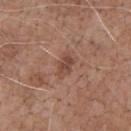Part of a total-body skin-imaging series; this lesion was reviewed on a skin check and was not flagged for biopsy.
Located on the chest.
The tile uses white-light illumination.
The total-body-photography lesion software estimated an area of roughly 4 mm², an outline eccentricity of about 0.8 (0 = round, 1 = elongated), and two-axis asymmetry of about 0.25. The software also gave a border-irregularity rating of about 2.5/10, a within-lesion color-variation index near 2.5/10, and radial color variation of about 1. And it measured a classifier nevus-likeness of about 20/100 and a lesion-detection confidence of about 100/100.
A male subject aged 68–72.
A roughly 15 mm field-of-view crop from a total-body skin photograph.
The recorded lesion diameter is about 3 mm.